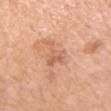No biopsy was performed on this lesion — it was imaged during a full skin examination and was not determined to be concerning.
From the chest.
The total-body-photography lesion software estimated a footprint of about 6 mm², an eccentricity of roughly 0.7, and two-axis asymmetry of about 0.65. It also reported a lesion color around L≈62 a*≈25 b*≈35 in CIELAB. The analysis additionally found a border-irregularity rating of about 7/10 and a peripheral color-asymmetry measure near 1. The analysis additionally found a nevus-likeness score of about 0/100 and a lesion-detection confidence of about 100/100.
A female subject, aged around 55.
Approximately 3.5 mm at its widest.
A 15 mm close-up tile from a total-body photography series done for melanoma screening.
Imaged with white-light lighting.